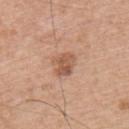{
  "biopsy_status": "not biopsied; imaged during a skin examination",
  "site": "back",
  "image": {
    "source": "total-body photography crop",
    "field_of_view_mm": 15
  },
  "lesion_size": {
    "long_diameter_mm_approx": 3.0
  },
  "lighting": "white-light",
  "automated_metrics": {
    "border_irregularity_0_10": 3.5,
    "peripheral_color_asymmetry": 1.5,
    "nevus_likeness_0_100": 20,
    "lesion_detection_confidence_0_100": 100
  },
  "patient": {
    "sex": "male",
    "age_approx": 55
  }
}